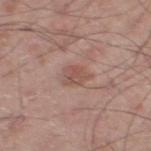The lesion was tiled from a total-body skin photograph and was not biopsied. A male patient, about 50 years old. The recorded lesion diameter is about 2.5 mm. A 15 mm close-up tile from a total-body photography series done for melanoma screening. Automated tile analysis of the lesion measured a footprint of about 4.5 mm², an outline eccentricity of about 0.4 (0 = round, 1 = elongated), and two-axis asymmetry of about 0.3. The software also gave about 8 CIELAB-L* units darker than the surrounding skin and a normalized border contrast of about 6. The analysis additionally found a classifier nevus-likeness of about 10/100 and a lesion-detection confidence of about 100/100. From the left thigh.